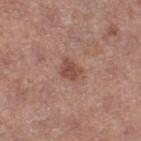The lesion was tiled from a total-body skin photograph and was not biopsied.
A 15 mm close-up extracted from a 3D total-body photography capture.
The tile uses white-light illumination.
A female subject, roughly 40 years of age.
Measured at roughly 3 mm in maximum diameter.
The lesion is located on the left thigh.